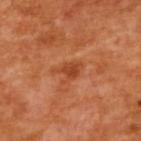Q: Was this lesion biopsied?
A: catalogued during a skin exam; not biopsied
Q: Patient demographics?
A: female, roughly 55 years of age
Q: Where on the body is the lesion?
A: the back
Q: What kind of image is this?
A: ~15 mm tile from a whole-body skin photo
Q: Illumination type?
A: cross-polarized illumination